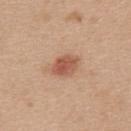<tbp_lesion>
<biopsy_status>not biopsied; imaged during a skin examination</biopsy_status>
<lesion_size>
  <long_diameter_mm_approx>3.5</long_diameter_mm_approx>
</lesion_size>
<site>upper back</site>
<lighting>white-light</lighting>
<image>
  <source>total-body photography crop</source>
  <field_of_view_mm>15</field_of_view_mm>
</image>
<automated_metrics>
  <color_variation_0_10>4.0</color_variation_0_10>
  <peripheral_color_asymmetry>1.5</peripheral_color_asymmetry>
  <nevus_likeness_0_100>95</nevus_likeness_0_100>
</automated_metrics>
<patient>
  <sex>female</sex>
  <age_approx>45</age_approx>
</patient>
</tbp_lesion>